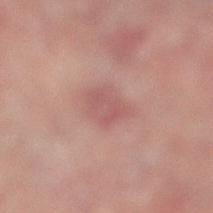Imaged during a routine full-body skin examination; the lesion was not biopsied and no histopathology is available.
Approximately 3.5 mm at its widest.
The tile uses cross-polarized illumination.
The total-body-photography lesion software estimated a lesion color around L≈49 a*≈21 b*≈20 in CIELAB, about 6 CIELAB-L* units darker than the surrounding skin, and a lesion-to-skin contrast of about 5 (normalized; higher = more distinct).
A male subject, about 60 years old.
From the right lower leg.
A 15 mm close-up tile from a total-body photography series done for melanoma screening.